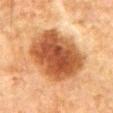Clinical impression:
Imaged during a routine full-body skin examination; the lesion was not biopsied and no histopathology is available.
Context:
Automated image analysis of the tile measured a lesion area of about 36 mm², an eccentricity of roughly 0.55, and a shape-asymmetry score of about 0.1 (0 = symmetric). It also reported a lesion color around L≈42 a*≈22 b*≈33 in CIELAB, roughly 15 lightness units darker than nearby skin, and a lesion-to-skin contrast of about 11 (normalized; higher = more distinct). And it measured border irregularity of about 1.5 on a 0–10 scale. A close-up tile cropped from a whole-body skin photograph, about 15 mm across. Captured under cross-polarized illumination. The lesion is located on the abdomen. The recorded lesion diameter is about 7 mm. A male subject approximately 80 years of age.